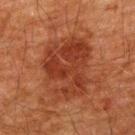Q: Was this lesion biopsied?
A: catalogued during a skin exam; not biopsied
Q: Illumination type?
A: cross-polarized illumination
Q: What did automated image analysis measure?
A: a lesion area of about 31 mm², an outline eccentricity of about 0.7 (0 = round, 1 = elongated), and a shape-asymmetry score of about 0.25 (0 = symmetric)
Q: What kind of image is this?
A: 15 mm crop, total-body photography
Q: How large is the lesion?
A: about 7.5 mm
Q: Patient demographics?
A: male, about 60 years old
Q: What is the anatomic site?
A: the upper back